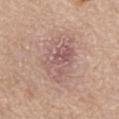biopsy_status: not biopsied; imaged during a skin examination
automated_metrics:
  area_mm2_approx: 18.0
  eccentricity: 0.75
  shape_asymmetry: 0.3
  cielab_L: 57
  cielab_a: 19
  cielab_b: 22
  vs_skin_contrast_norm: 6.0
  color_variation_0_10: 6.0
  peripheral_color_asymmetry: 2.0
  nevus_likeness_0_100: 0
  lesion_detection_confidence_0_100: 100
lighting: white-light
image:
  source: total-body photography crop
  field_of_view_mm: 15
lesion_size:
  long_diameter_mm_approx: 6.0
site: abdomen
patient:
  sex: male
  age_approx: 65